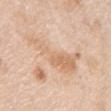Case summary:
* anatomic site · the right upper arm
* image · total-body-photography crop, ~15 mm field of view
* automated metrics · an area of roughly 22 mm², an outline eccentricity of about 0.9 (0 = round, 1 = elongated), and a symmetry-axis asymmetry near 0.5; an automated nevus-likeness rating near 0 out of 100 and a detector confidence of about 75 out of 100 that the crop contains a lesion
* subject · female, aged 43 to 47